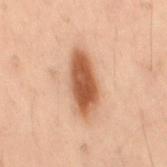The lesion is on the back.
A 15 mm crop from a total-body photograph taken for skin-cancer surveillance.
A female subject, aged 53–57.
Captured under cross-polarized illumination.
Measured at roughly 6.5 mm in maximum diameter.